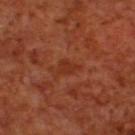<record>
  <biopsy_status>not biopsied; imaged during a skin examination</biopsy_status>
  <image>
    <source>total-body photography crop</source>
    <field_of_view_mm>15</field_of_view_mm>
  </image>
  <site>back</site>
  <lesion_size>
    <long_diameter_mm_approx>2.5</long_diameter_mm_approx>
  </lesion_size>
  <patient>
    <sex>male</sex>
    <age_approx>70</age_approx>
  </patient>
  <lighting>cross-polarized</lighting>
  <automated_metrics>
    <cielab_L>32</cielab_L>
    <cielab_a>28</cielab_a>
    <cielab_b>33</cielab_b>
    <vs_skin_darker_L>6.0</vs_skin_darker_L>
    <vs_skin_contrast_norm>5.5</vs_skin_contrast_norm>
    <border_irregularity_0_10>5.5</border_irregularity_0_10>
    <color_variation_0_10>1.0</color_variation_0_10>
    <peripheral_color_asymmetry>0.5</peripheral_color_asymmetry>
    <lesion_detection_confidence_0_100>100</lesion_detection_confidence_0_100>
  </automated_metrics>
</record>